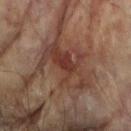Impression: The lesion was tiled from a total-body skin photograph and was not biopsied. Context: A female subject, aged approximately 80. A 15 mm crop from a total-body photograph taken for skin-cancer surveillance. The tile uses cross-polarized illumination. The lesion is located on the left arm. Approximately 6 mm at its widest.